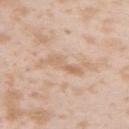Case summary:
- workup · total-body-photography surveillance lesion; no biopsy
- subject · female, roughly 25 years of age
- acquisition · ~15 mm crop, total-body skin-cancer survey
- image-analysis metrics · a footprint of about 7 mm² and an outline eccentricity of about 0.95 (0 = round, 1 = elongated); border irregularity of about 8 on a 0–10 scale, a within-lesion color-variation index near 4/10, and radial color variation of about 1.5; an automated nevus-likeness rating near 0 out of 100
- lighting · white-light
- anatomic site · the left upper arm